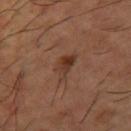The lesion is on the leg.
The total-body-photography lesion software estimated a footprint of about 5 mm², an eccentricity of roughly 0.85, and a symmetry-axis asymmetry near 0.45. And it measured a nevus-likeness score of about 65/100 and lesion-presence confidence of about 100/100.
Longest diameter approximately 3.5 mm.
A male patient aged approximately 65.
A 15 mm close-up extracted from a 3D total-body photography capture.